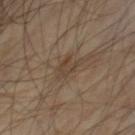{"biopsy_status": "not biopsied; imaged during a skin examination", "automated_metrics": {"area_mm2_approx": 4.5, "eccentricity": 0.65, "shape_asymmetry": 0.45, "vs_skin_darker_L": 6.0, "vs_skin_contrast_norm": 5.5, "lesion_detection_confidence_0_100": 55}, "patient": {"sex": "male", "age_approx": 70}, "site": "chest", "image": {"source": "total-body photography crop", "field_of_view_mm": 15}, "lighting": "cross-polarized"}